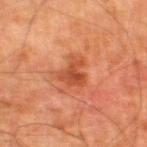<tbp_lesion>
<biopsy_status>not biopsied; imaged during a skin examination</biopsy_status>
<site>left thigh</site>
<patient>
  <sex>male</sex>
  <age_approx>80</age_approx>
</patient>
<image>
  <source>total-body photography crop</source>
  <field_of_view_mm>15</field_of_view_mm>
</image>
<lighting>cross-polarized</lighting>
<lesion_size>
  <long_diameter_mm_approx>4.0</long_diameter_mm_approx>
</lesion_size>
</tbp_lesion>